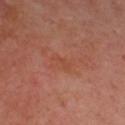An algorithmic analysis of the crop reported an area of roughly 2.5 mm², an outline eccentricity of about 0.95 (0 = round, 1 = elongated), and a shape-asymmetry score of about 0.5 (0 = symmetric). It also reported an average lesion color of about L≈45 a*≈27 b*≈32 (CIELAB), roughly 5 lightness units darker than nearby skin, and a normalized border contrast of about 5. It also reported a detector confidence of about 100 out of 100 that the crop contains a lesion. A region of skin cropped from a whole-body photographic capture, roughly 15 mm wide. The patient is a male aged 48 to 52. On the upper back. The lesion's longest dimension is about 2.5 mm.